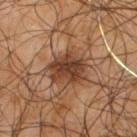The lesion was tiled from a total-body skin photograph and was not biopsied.
Cropped from a total-body skin-imaging series; the visible field is about 15 mm.
Captured under cross-polarized illumination.
The subject is a male roughly 65 years of age.
From the upper back.